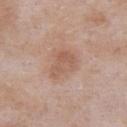Context: A region of skin cropped from a whole-body photographic capture, roughly 15 mm wide. A male subject, aged 53 to 57. The recorded lesion diameter is about 3.5 mm. The tile uses white-light illumination. Automated tile analysis of the lesion measured an area of roughly 7.5 mm² and two-axis asymmetry of about 0.35. It also reported an average lesion color of about L≈57 a*≈20 b*≈28 (CIELAB), roughly 7 lightness units darker than nearby skin, and a normalized border contrast of about 5.5. The software also gave an automated nevus-likeness rating near 10 out of 100 and a detector confidence of about 100 out of 100 that the crop contains a lesion. On the front of the torso.